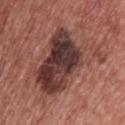On biopsy, histopathology showed a dysplastic (Clark) nevus.